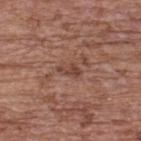follow-up: imaged on a skin check; not biopsied | acquisition: 15 mm crop, total-body photography | patient: female, about 65 years old | body site: the upper back.